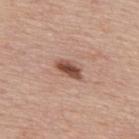Q: Is there a histopathology result?
A: imaged on a skin check; not biopsied
Q: What lighting was used for the tile?
A: white-light illumination
Q: Who is the patient?
A: male, in their mid-70s
Q: Where on the body is the lesion?
A: the upper back
Q: What kind of image is this?
A: ~15 mm tile from a whole-body skin photo